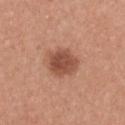notes: total-body-photography surveillance lesion; no biopsy
location: the chest
lesion diameter: ≈4 mm
subject: female, aged 38 to 42
image source: 15 mm crop, total-body photography
lighting: white-light illumination